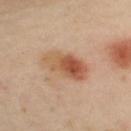Assessment:
The lesion was tiled from a total-body skin photograph and was not biopsied.
Clinical summary:
A 15 mm crop from a total-body photograph taken for skin-cancer surveillance. Located on the left upper arm. The total-body-photography lesion software estimated a mean CIELAB color near L≈57 a*≈21 b*≈34, a lesion–skin lightness drop of about 11, and a normalized lesion–skin contrast near 8. And it measured a nevus-likeness score of about 100/100. The subject is a female aged around 40. The tile uses cross-polarized illumination.